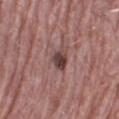– follow-up: catalogued during a skin exam; not biopsied
– image source: 15 mm crop, total-body photography
– TBP lesion metrics: a shape eccentricity near 0.55 and a shape-asymmetry score of about 0.3 (0 = symmetric)
– location: the right thigh
– lesion diameter: about 2.5 mm
– subject: female, roughly 70 years of age